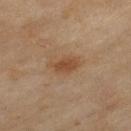| feature | finding |
|---|---|
| site | the left thigh |
| diameter | ~4 mm (longest diameter) |
| subject | female, aged 58–62 |
| imaging modality | total-body-photography crop, ~15 mm field of view |
| lighting | cross-polarized illumination |